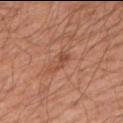The lesion was photographed on a routine skin check and not biopsied; there is no pathology result. A male subject aged 63–67. From the left upper arm. The lesion's longest dimension is about 3 mm. Captured under white-light illumination. A 15 mm close-up tile from a total-body photography series done for melanoma screening.